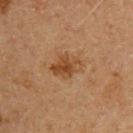| field | value |
|---|---|
| follow-up | catalogued during a skin exam; not biopsied |
| subject | male, aged 58–62 |
| lighting | cross-polarized illumination |
| image | 15 mm crop, total-body photography |